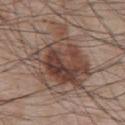• biopsy status — total-body-photography surveillance lesion; no biopsy
• automated lesion analysis — an area of roughly 35 mm², a shape eccentricity near 0.35, and two-axis asymmetry of about 0.45; a lesion color around L≈44 a*≈17 b*≈24 in CIELAB, a lesion–skin lightness drop of about 11, and a normalized lesion–skin contrast near 8.5; a classifier nevus-likeness of about 75/100 and a detector confidence of about 100 out of 100 that the crop contains a lesion
• subject — male, in their mid-60s
• lesion diameter — about 8 mm
• site — the back
• image source — 15 mm crop, total-body photography
• illumination — white-light